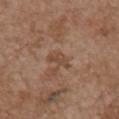• workup: no biopsy performed (imaged during a skin exam)
• size: about 3 mm
• body site: the chest
• subject: female, aged 63–67
• imaging modality: ~15 mm crop, total-body skin-cancer survey
• automated lesion analysis: an average lesion color of about L≈46 a*≈18 b*≈30 (CIELAB), roughly 7 lightness units darker than nearby skin, and a normalized lesion–skin contrast near 6